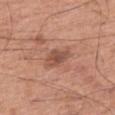| field | value |
|---|---|
| biopsy status | imaged on a skin check; not biopsied |
| location | the right thigh |
| lesion size | ≈3 mm |
| patient | male, approximately 65 years of age |
| image source | ~15 mm tile from a whole-body skin photo |